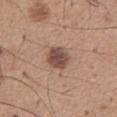| feature | finding |
|---|---|
| workup | imaged on a skin check; not biopsied |
| body site | the chest |
| image source | 15 mm crop, total-body photography |
| subject | male, aged 63–67 |
| size | ≈3 mm |
| automated lesion analysis | a nevus-likeness score of about 65/100 and a detector confidence of about 100 out of 100 that the crop contains a lesion |